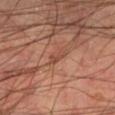Clinical impression: This lesion was catalogued during total-body skin photography and was not selected for biopsy. Clinical summary: From the left lower leg. Cropped from a total-body skin-imaging series; the visible field is about 15 mm. A male subject roughly 45 years of age. The tile uses cross-polarized illumination.